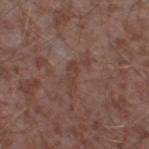No biopsy was performed on this lesion — it was imaged during a full skin examination and was not determined to be concerning. This image is a 15 mm lesion crop taken from a total-body photograph. About 4.5 mm across. The lesion is located on the right thigh. A male patient roughly 45 years of age. This is a white-light tile.